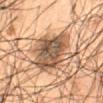Captured during whole-body skin photography for melanoma surveillance; the lesion was not biopsied. The total-body-photography lesion software estimated border irregularity of about 3.5 on a 0–10 scale, a color-variation rating of about 8/10, and a peripheral color-asymmetry measure near 2.5. And it measured a classifier nevus-likeness of about 20/100 and a lesion-detection confidence of about 80/100. A 15 mm close-up tile from a total-body photography series done for melanoma screening. Captured under cross-polarized illumination. About 6 mm across. The subject is a male approximately 55 years of age. The lesion is located on the abdomen.